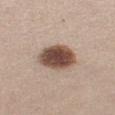Captured during whole-body skin photography for melanoma surveillance; the lesion was not biopsied. A female subject, approximately 25 years of age. A 15 mm crop from a total-body photograph taken for skin-cancer surveillance. The lesion is on the left thigh. Captured under white-light illumination.